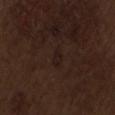<lesion>
<image>
  <source>total-body photography crop</source>
  <field_of_view_mm>15</field_of_view_mm>
</image>
<lesion_size>
  <long_diameter_mm_approx>3.0</long_diameter_mm_approx>
</lesion_size>
<patient>
  <sex>male</sex>
  <age_approx>70</age_approx>
</patient>
<lighting>white-light</lighting>
<site>lower back</site>
<automated_metrics>
  <cielab_L>17</cielab_L>
  <cielab_a>13</cielab_a>
  <cielab_b>17</cielab_b>
  <vs_skin_darker_L>3.0</vs_skin_darker_L>
  <vs_skin_contrast_norm>5.0</vs_skin_contrast_norm>
  <border_irregularity_0_10>4.0</border_irregularity_0_10>
  <color_variation_0_10>1.0</color_variation_0_10>
</automated_metrics>
</lesion>